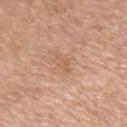Q: Was this lesion biopsied?
A: total-body-photography surveillance lesion; no biopsy
Q: How was this image acquired?
A: total-body-photography crop, ~15 mm field of view
Q: What are the patient's age and sex?
A: male, aged 73 to 77
Q: Where on the body is the lesion?
A: the left upper arm